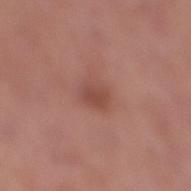Part of a total-body skin-imaging series; this lesion was reviewed on a skin check and was not flagged for biopsy. A close-up tile cropped from a whole-body skin photograph, about 15 mm across. The lesion is located on the right lower leg. A female subject, aged 58 to 62. Measured at roughly 2.5 mm in maximum diameter. This is a white-light tile.